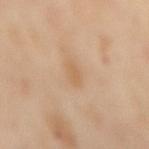workup = catalogued during a skin exam; not biopsied | automated lesion analysis = a border-irregularity index near 2/10; a lesion-detection confidence of about 100/100 | patient = female, aged approximately 55 | lighting = cross-polarized illumination | anatomic site = the mid back | acquisition = ~15 mm crop, total-body skin-cancer survey.